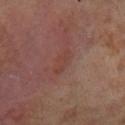Clinical summary: A lesion tile, about 15 mm wide, cut from a 3D total-body photograph. The lesion is on the left lower leg. Approximately 3.5 mm at its widest. A male patient aged 68–72. Captured under cross-polarized illumination. Automated image analysis of the tile measured a shape-asymmetry score of about 0.3 (0 = symmetric). The analysis additionally found a border-irregularity index near 3/10, a within-lesion color-variation index near 2/10, and radial color variation of about 0.5.